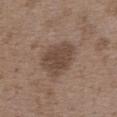No biopsy was performed on this lesion — it was imaged during a full skin examination and was not determined to be concerning. The lesion's longest dimension is about 5 mm. A female subject, aged approximately 35. The tile uses white-light illumination. A lesion tile, about 15 mm wide, cut from a 3D total-body photograph. Automated tile analysis of the lesion measured roughly 10 lightness units darker than nearby skin and a normalized lesion–skin contrast near 7.5. The software also gave a border-irregularity index near 2/10, internal color variation of about 2.5 on a 0–10 scale, and a peripheral color-asymmetry measure near 1. The analysis additionally found an automated nevus-likeness rating near 10 out of 100 and lesion-presence confidence of about 100/100. From the upper back.